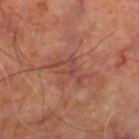biopsy status: no biopsy performed (imaged during a skin exam); acquisition: total-body-photography crop, ~15 mm field of view; location: the left thigh; patient: aged 63 to 67; automated metrics: a footprint of about 5.5 mm² and a shape-asymmetry score of about 0.55 (0 = symmetric); lighting: cross-polarized illumination; lesion size: about 4 mm.